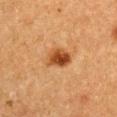follow-up = total-body-photography surveillance lesion; no biopsy
subject = male, roughly 75 years of age
lesion diameter = about 3 mm
imaging modality = total-body-photography crop, ~15 mm field of view
anatomic site = the right upper arm
image-analysis metrics = a shape eccentricity near 0.65 and two-axis asymmetry of about 0.3; a border-irregularity rating of about 2.5/10, a color-variation rating of about 5/10, and a peripheral color-asymmetry measure near 2; a nevus-likeness score of about 95/100 and lesion-presence confidence of about 100/100
lighting = cross-polarized